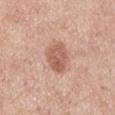Notes:
- workup: total-body-photography surveillance lesion; no biopsy
- location: the back
- subject: male, aged 53 to 57
- imaging modality: 15 mm crop, total-body photography
- automated metrics: a footprint of about 9.5 mm², an outline eccentricity of about 0.8 (0 = round, 1 = elongated), and two-axis asymmetry of about 0.25; border irregularity of about 2.5 on a 0–10 scale, a within-lesion color-variation index near 3.5/10, and peripheral color asymmetry of about 1.5; a detector confidence of about 100 out of 100 that the crop contains a lesion
- lesion diameter: ≈4.5 mm